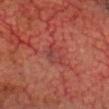biopsy status — imaged on a skin check; not biopsied | body site — the head or neck | size — ~3 mm (longest diameter) | automated lesion analysis — an area of roughly 5 mm², a shape eccentricity near 0.75, and two-axis asymmetry of about 0.3; a mean CIELAB color near L≈34 a*≈26 b*≈22, a lesion–skin lightness drop of about 5, and a normalized border contrast of about 5; border irregularity of about 3 on a 0–10 scale, a color-variation rating of about 4/10, and a peripheral color-asymmetry measure near 1; lesion-presence confidence of about 90/100 | lighting — cross-polarized illumination | image — 15 mm crop, total-body photography | patient — male, aged around 75.